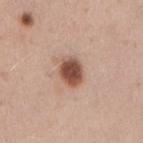Part of a total-body skin-imaging series; this lesion was reviewed on a skin check and was not flagged for biopsy.
Longest diameter approximately 3.5 mm.
A male patient, in their mid- to late 30s.
On the right upper arm.
This image is a 15 mm lesion crop taken from a total-body photograph.